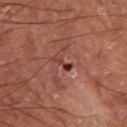lighting: cross-polarized
lesion_size:
  long_diameter_mm_approx: 2.5
image:
  source: total-body photography crop
  field_of_view_mm: 15
automated_metrics:
  eccentricity: 0.95
  shape_asymmetry: 0.5
  cielab_L: 32
  cielab_a: 25
  cielab_b: 22
  vs_skin_darker_L: 10.0
  vs_skin_contrast_norm: 9.0
  border_irregularity_0_10: 5.5
  color_variation_0_10: 0.0
  peripheral_color_asymmetry: 0.0
  nevus_likeness_0_100: 0
  lesion_detection_confidence_0_100: 95
patient:
  sex: male
  age_approx: 55
site: left thigh